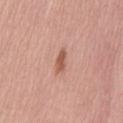Impression:
The lesion was tiled from a total-body skin photograph and was not biopsied.
Image and clinical context:
An algorithmic analysis of the crop reported an eccentricity of roughly 0.9 and a symmetry-axis asymmetry near 0.3. The analysis additionally found a border-irregularity index near 3/10, a within-lesion color-variation index near 1.5/10, and a peripheral color-asymmetry measure near 0.5. And it measured lesion-presence confidence of about 100/100. A lesion tile, about 15 mm wide, cut from a 3D total-body photograph. This is a white-light tile. About 2.5 mm across. A male subject in their 70s. From the mid back.